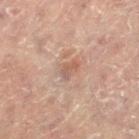Q: Was this lesion biopsied?
A: no biopsy performed (imaged during a skin exam)
Q: Illumination type?
A: cross-polarized
Q: Who is the patient?
A: female, about 80 years old
Q: What is the imaging modality?
A: total-body-photography crop, ~15 mm field of view
Q: Lesion location?
A: the right leg